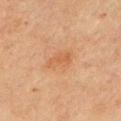<lesion>
<biopsy_status>not biopsied; imaged during a skin examination</biopsy_status>
<site>right upper arm</site>
<patient>
  <sex>male</sex>
  <age_approx>65</age_approx>
</patient>
<lesion_size>
  <long_diameter_mm_approx>3.5</long_diameter_mm_approx>
</lesion_size>
<image>
  <source>total-body photography crop</source>
  <field_of_view_mm>15</field_of_view_mm>
</image>
<automated_metrics>
  <cielab_L>48</cielab_L>
  <cielab_a>19</cielab_a>
  <cielab_b>32</cielab_b>
  <vs_skin_contrast_norm>5.0</vs_skin_contrast_norm>
  <nevus_likeness_0_100>0</nevus_likeness_0_100>
  <lesion_detection_confidence_0_100>100</lesion_detection_confidence_0_100>
</automated_metrics>
</lesion>